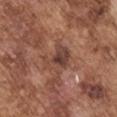The tile uses white-light illumination. On the left upper arm. About 3.5 mm across. A close-up tile cropped from a whole-body skin photograph, about 15 mm across. The subject is a male aged 73 to 77. Automated tile analysis of the lesion measured a border-irregularity index near 5/10, internal color variation of about 5.5 on a 0–10 scale, and peripheral color asymmetry of about 1.5. It also reported an automated nevus-likeness rating near 0 out of 100 and a detector confidence of about 95 out of 100 that the crop contains a lesion.